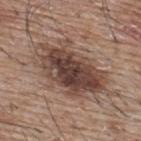• workup · catalogued during a skin exam; not biopsied
• size · ≈8.5 mm
• lighting · white-light
• image source · total-body-photography crop, ~15 mm field of view
• automated lesion analysis · a classifier nevus-likeness of about 45/100 and a lesion-detection confidence of about 100/100
• anatomic site · the back
• subject · male, in their mid-60s